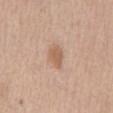Impression: No biopsy was performed on this lesion — it was imaged during a full skin examination and was not determined to be concerning. Acquisition and patient details: This is a white-light tile. Longest diameter approximately 3 mm. A close-up tile cropped from a whole-body skin photograph, about 15 mm across. From the abdomen. A male patient aged approximately 75.